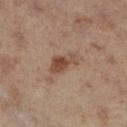Assessment: Imaged during a routine full-body skin examination; the lesion was not biopsied and no histopathology is available. Acquisition and patient details: A 15 mm close-up extracted from a 3D total-body photography capture. On the right lower leg. A female subject aged 38 to 42. Imaged with cross-polarized lighting.